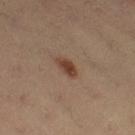The lesion was tiled from a total-body skin photograph and was not biopsied. A roughly 15 mm field-of-view crop from a total-body skin photograph. The lesion is on the right thigh. A female subject approximately 35 years of age.